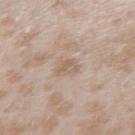Clinical impression:
The lesion was photographed on a routine skin check and not biopsied; there is no pathology result.
Image and clinical context:
The recorded lesion diameter is about 2.5 mm. The lesion is located on the left forearm. Captured under white-light illumination. A female patient about 25 years old. A region of skin cropped from a whole-body photographic capture, roughly 15 mm wide.